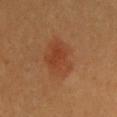Imaged during a routine full-body skin examination; the lesion was not biopsied and no histopathology is available.
A close-up tile cropped from a whole-body skin photograph, about 15 mm across.
A female subject, aged 28 to 32.
Measured at roughly 5 mm in maximum diameter.
The tile uses cross-polarized illumination.
Located on the chest.